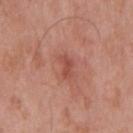Assessment: Recorded during total-body skin imaging; not selected for excision or biopsy. Clinical summary: The subject is a male aged 53 to 57. Imaged with white-light lighting. An algorithmic analysis of the crop reported a lesion color around L≈50 a*≈28 b*≈29 in CIELAB, a lesion–skin lightness drop of about 9, and a lesion-to-skin contrast of about 6 (normalized; higher = more distinct). The software also gave internal color variation of about 0.5 on a 0–10 scale and peripheral color asymmetry of about 0. It also reported a classifier nevus-likeness of about 5/100 and a lesion-detection confidence of about 100/100. Located on the mid back. About 2.5 mm across. A 15 mm close-up tile from a total-body photography series done for melanoma screening.